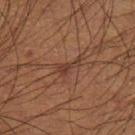Imaged during a routine full-body skin examination; the lesion was not biopsied and no histopathology is available. Imaged with cross-polarized lighting. Cropped from a total-body skin-imaging series; the visible field is about 15 mm. About 3 mm across. The lesion-visualizer software estimated a border-irregularity rating of about 6/10, a color-variation rating of about 1.5/10, and a peripheral color-asymmetry measure near 0.5. It also reported a nevus-likeness score of about 0/100 and a lesion-detection confidence of about 95/100. The patient is a male aged 58 to 62.